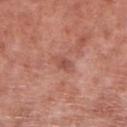biopsy status = catalogued during a skin exam; not biopsied
imaging modality = ~15 mm tile from a whole-body skin photo
tile lighting = white-light
patient = male, in their mid- to late 50s
site = the right lower leg
diameter = ≈2.5 mm